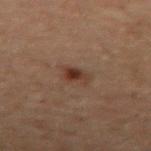Findings:
– notes: catalogued during a skin exam; not biopsied
– acquisition: ~15 mm crop, total-body skin-cancer survey
– lesion size: ≈3 mm
– TBP lesion metrics: a footprint of about 4 mm², a shape eccentricity near 0.8, and two-axis asymmetry of about 0.35; a lesion color around L≈26 a*≈15 b*≈21 in CIELAB, roughly 8 lightness units darker than nearby skin, and a normalized lesion–skin contrast near 8.5; a border-irregularity index near 3.5/10 and internal color variation of about 4 on a 0–10 scale; an automated nevus-likeness rating near 90 out of 100 and lesion-presence confidence of about 100/100
– anatomic site: the right thigh
– subject: male, aged 68–72